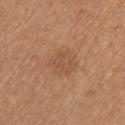Q: Patient demographics?
A: female, approximately 45 years of age
Q: What is the imaging modality?
A: total-body-photography crop, ~15 mm field of view
Q: Where on the body is the lesion?
A: the right upper arm
Q: How was the tile lit?
A: white-light